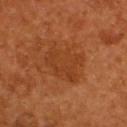Q: Was this lesion biopsied?
A: total-body-photography surveillance lesion; no biopsy
Q: Where on the body is the lesion?
A: the upper back
Q: Illumination type?
A: cross-polarized
Q: What are the patient's age and sex?
A: male, aged 53–57
Q: How large is the lesion?
A: about 5 mm
Q: What is the imaging modality?
A: 15 mm crop, total-body photography
Q: What did automated image analysis measure?
A: an area of roughly 15 mm², an eccentricity of roughly 0.75, and a symmetry-axis asymmetry near 0.25; a lesion color around L≈36 a*≈25 b*≈36 in CIELAB, roughly 6 lightness units darker than nearby skin, and a normalized border contrast of about 5; a within-lesion color-variation index near 2.5/10 and peripheral color asymmetry of about 1